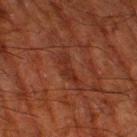Findings:
• notes · no biopsy performed (imaged during a skin exam)
• imaging modality · ~15 mm tile from a whole-body skin photo
• site · the leg
• size · ~4 mm (longest diameter)
• TBP lesion metrics · a lesion area of about 5 mm² and an eccentricity of roughly 0.9; a lesion color around L≈23 a*≈21 b*≈24 in CIELAB; a classifier nevus-likeness of about 0/100
• patient · male, approximately 80 years of age